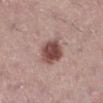{
  "lighting": "white-light",
  "site": "right lower leg",
  "image": {
    "source": "total-body photography crop",
    "field_of_view_mm": 15
  },
  "automated_metrics": {
    "area_mm2_approx": 10.0,
    "eccentricity": 0.2,
    "shape_asymmetry": 0.15,
    "cielab_L": 48,
    "cielab_a": 21,
    "cielab_b": 22,
    "vs_skin_darker_L": 15.0,
    "vs_skin_contrast_norm": 10.5
  },
  "patient": {
    "sex": "female",
    "age_approx": 40
  }
}